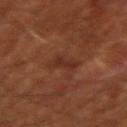Impression:
The lesion was tiled from a total-body skin photograph and was not biopsied.
Clinical summary:
The subject is a male in their mid- to late 60s. This is a cross-polarized tile. The lesion is on the right upper arm. An algorithmic analysis of the crop reported a footprint of about 4.5 mm² and a symmetry-axis asymmetry near 0.3. Measured at roughly 3 mm in maximum diameter. A roughly 15 mm field-of-view crop from a total-body skin photograph.